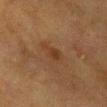Q: Is there a histopathology result?
A: total-body-photography surveillance lesion; no biopsy
Q: How was this image acquired?
A: total-body-photography crop, ~15 mm field of view
Q: What is the anatomic site?
A: the chest
Q: Patient demographics?
A: female, aged 53–57